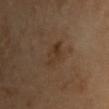A 15 mm crop from a total-body photograph taken for skin-cancer surveillance. The patient is a male aged 53–57. Captured under cross-polarized illumination. The lesion is on the chest. Automated image analysis of the tile measured an area of roughly 6 mm², an eccentricity of roughly 0.7, and a shape-asymmetry score of about 0.2 (0 = symmetric). It also reported border irregularity of about 2.5 on a 0–10 scale and a within-lesion color-variation index near 4.5/10.